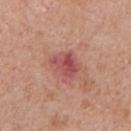Captured during whole-body skin photography for melanoma surveillance; the lesion was not biopsied.